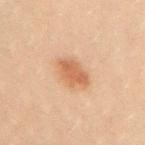The lesion was photographed on a routine skin check and not biopsied; there is no pathology result. Imaged with cross-polarized lighting. A close-up tile cropped from a whole-body skin photograph, about 15 mm across. A female patient, about 20 years old. The lesion is located on the chest.